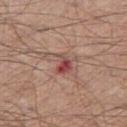{"biopsy_status": "not biopsied; imaged during a skin examination", "patient": {"sex": "male", "age_approx": 65}, "site": "right thigh", "image": {"source": "total-body photography crop", "field_of_view_mm": 15}}